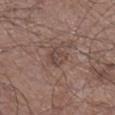<tbp_lesion>
  <biopsy_status>not biopsied; imaged during a skin examination</biopsy_status>
  <image>
    <source>total-body photography crop</source>
    <field_of_view_mm>15</field_of_view_mm>
  </image>
  <automated_metrics>
    <nevus_likeness_0_100>0</nevus_likeness_0_100>
    <lesion_detection_confidence_0_100>100</lesion_detection_confidence_0_100>
  </automated_metrics>
  <lesion_size>
    <long_diameter_mm_approx>2.5</long_diameter_mm_approx>
  </lesion_size>
  <site>right lower leg</site>
  <lighting>white-light</lighting>
  <patient>
    <sex>male</sex>
    <age_approx>60</age_approx>
  </patient>
</tbp_lesion>